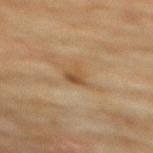workup: no biopsy performed (imaged during a skin exam) | tile lighting: cross-polarized illumination | patient: female, aged 78–82 | lesion diameter: ≈2.5 mm | automated metrics: a lesion area of about 4 mm² and an outline eccentricity of about 0.5 (0 = round, 1 = elongated); a border-irregularity index near 4/10, internal color variation of about 3.5 on a 0–10 scale, and a peripheral color-asymmetry measure near 1.5 | image source: ~15 mm crop, total-body skin-cancer survey | location: the upper back.